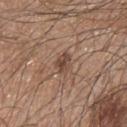This lesion was catalogued during total-body skin photography and was not selected for biopsy.
The lesion is located on the upper back.
A 15 mm close-up tile from a total-body photography series done for melanoma screening.
The patient is a male in their mid- to late 40s.
The recorded lesion diameter is about 3 mm.
The tile uses white-light illumination.